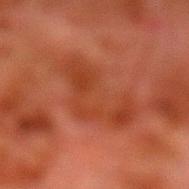Recorded during total-body skin imaging; not selected for excision or biopsy. The subject is a male aged 78–82. On the left lower leg. A 15 mm close-up extracted from a 3D total-body photography capture.